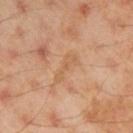Q: Is there a histopathology result?
A: total-body-photography surveillance lesion; no biopsy
Q: Illumination type?
A: cross-polarized
Q: What are the patient's age and sex?
A: male, about 45 years old
Q: What is the lesion's diameter?
A: about 4 mm
Q: Lesion location?
A: the right upper arm
Q: What is the imaging modality?
A: total-body-photography crop, ~15 mm field of view